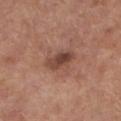{
  "biopsy_status": "not biopsied; imaged during a skin examination",
  "image": {
    "source": "total-body photography crop",
    "field_of_view_mm": 15
  },
  "site": "left lower leg",
  "patient": {
    "sex": "male",
    "age_approx": 70
  }
}